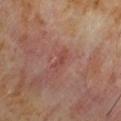<lesion>
<biopsy_status>not biopsied; imaged during a skin examination</biopsy_status>
<lighting>cross-polarized</lighting>
<lesion_size>
  <long_diameter_mm_approx>3.0</long_diameter_mm_approx>
</lesion_size>
<site>right upper arm</site>
<image>
  <source>total-body photography crop</source>
  <field_of_view_mm>15</field_of_view_mm>
</image>
<patient>
  <sex>male</sex>
  <age_approx>60</age_approx>
</patient>
</lesion>